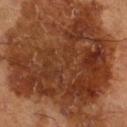The lesion was tiled from a total-body skin photograph and was not biopsied.
A male patient approximately 65 years of age.
On the right lower leg.
A 15 mm close-up tile from a total-body photography series done for melanoma screening.
Automated image analysis of the tile measured a nevus-likeness score of about 0/100 and lesion-presence confidence of about 100/100.
Captured under cross-polarized illumination.
Measured at roughly 13.5 mm in maximum diameter.